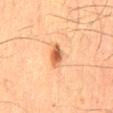Q: Was this lesion biopsied?
A: total-body-photography surveillance lesion; no biopsy
Q: Who is the patient?
A: male, approximately 65 years of age
Q: What is the imaging modality?
A: total-body-photography crop, ~15 mm field of view
Q: What is the anatomic site?
A: the back